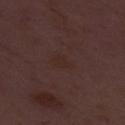workup: catalogued during a skin exam; not biopsied | subject: male, in their 50s | acquisition: ~15 mm tile from a whole-body skin photo | automated metrics: an eccentricity of roughly 0.8 and a shape-asymmetry score of about 0.3 (0 = symmetric); a border-irregularity index near 3/10, a color-variation rating of about 1/10, and radial color variation of about 0.5 | diameter: ~3 mm (longest diameter).